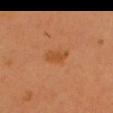Assessment:
This lesion was catalogued during total-body skin photography and was not selected for biopsy.
Context:
The lesion-visualizer software estimated a classifier nevus-likeness of about 25/100. The tile uses cross-polarized illumination. On the head or neck. About 3 mm across. Cropped from a whole-body photographic skin survey; the tile spans about 15 mm. The patient is a male aged around 60.